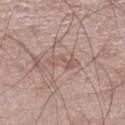  biopsy_status: not biopsied; imaged during a skin examination
  site: left lower leg
  image:
    source: total-body photography crop
    field_of_view_mm: 15
  patient:
    sex: male
    age_approx: 60
  automated_metrics:
    cielab_L: 56
    cielab_a: 19
    cielab_b: 25
    vs_skin_darker_L: 7.0
    vs_skin_contrast_norm: 5.0